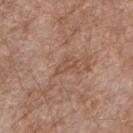Impression:
No biopsy was performed on this lesion — it was imaged during a full skin examination and was not determined to be concerning.
Clinical summary:
On the right forearm. A 15 mm crop from a total-body photograph taken for skin-cancer surveillance. A male subject, roughly 55 years of age. Imaged with white-light lighting. Approximately 2.5 mm at its widest. The total-body-photography lesion software estimated a border-irregularity index near 6.5/10 and internal color variation of about 0 on a 0–10 scale.